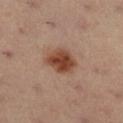Clinical impression: Imaged during a routine full-body skin examination; the lesion was not biopsied and no histopathology is available. Acquisition and patient details: Located on the right lower leg. This image is a 15 mm lesion crop taken from a total-body photograph. A male patient aged approximately 55. The lesion's longest dimension is about 3.5 mm. The tile uses cross-polarized illumination.